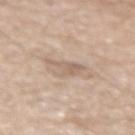Clinical impression: Imaged during a routine full-body skin examination; the lesion was not biopsied and no histopathology is available. Clinical summary: The lesion is on the arm. A 15 mm crop from a total-body photograph taken for skin-cancer surveillance. Approximately 3.5 mm at its widest. A male patient, approximately 70 years of age. Captured under white-light illumination.